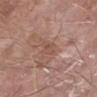| feature | finding |
|---|---|
| follow-up | no biopsy performed (imaged during a skin exam) |
| image | 15 mm crop, total-body photography |
| diameter | ≈3 mm |
| patient | male, aged approximately 55 |
| illumination | white-light |
| anatomic site | the left lower leg |